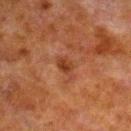Part of a total-body skin-imaging series; this lesion was reviewed on a skin check and was not flagged for biopsy. Cropped from a total-body skin-imaging series; the visible field is about 15 mm. On the leg. A male patient aged around 80.